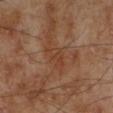Captured during whole-body skin photography for melanoma surveillance; the lesion was not biopsied.
A region of skin cropped from a whole-body photographic capture, roughly 15 mm wide.
The lesion is on the left lower leg.
The total-body-photography lesion software estimated an area of roughly 5 mm², a shape eccentricity near 0.85, and a symmetry-axis asymmetry near 0.4. It also reported a border-irregularity index near 4/10, a within-lesion color-variation index near 2/10, and radial color variation of about 0.5.
Imaged with cross-polarized lighting.
A male subject aged 68–72.